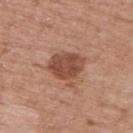Findings:
- biopsy status — imaged on a skin check; not biopsied
- image-analysis metrics — a mean CIELAB color near L≈48 a*≈23 b*≈30, a lesion–skin lightness drop of about 12, and a normalized border contrast of about 9; an automated nevus-likeness rating near 60 out of 100 and lesion-presence confidence of about 100/100
- subject — male, roughly 80 years of age
- site — the upper back
- lesion size — ~4 mm (longest diameter)
- acquisition — 15 mm crop, total-body photography